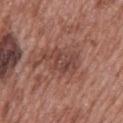Findings:
• workup — total-body-photography surveillance lesion; no biopsy
• subject — female, roughly 60 years of age
• size — about 5 mm
• automated lesion analysis — an area of roughly 11 mm² and an eccentricity of roughly 0.75
• imaging modality — 15 mm crop, total-body photography
• illumination — white-light
• anatomic site — the back